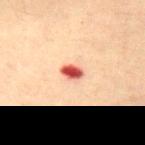Notes:
- image: total-body-photography crop, ~15 mm field of view
- lighting: cross-polarized
- size: about 2.5 mm
- patient: female, in their 80s
- body site: the lower back